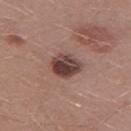The lesion was tiled from a total-body skin photograph and was not biopsied. Approximately 4 mm at its widest. From the left thigh. A male patient, approximately 30 years of age. Cropped from a total-body skin-imaging series; the visible field is about 15 mm. The lesion-visualizer software estimated a lesion area of about 9 mm², an eccentricity of roughly 0.6, and two-axis asymmetry of about 0.15.